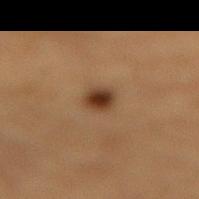* follow-up · no biopsy performed (imaged during a skin exam)
* patient · male, aged around 85 or older
* diameter · ~2.5 mm (longest diameter)
* tile lighting · cross-polarized illumination
* body site · the lower back
* imaging modality · total-body-photography crop, ~15 mm field of view